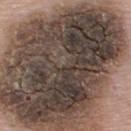Impression:
The lesion was tiled from a total-body skin photograph and was not biopsied.
Background:
A close-up tile cropped from a whole-body skin photograph, about 15 mm across. On the upper back. A male subject aged 53–57. The lesion's longest dimension is about 14.5 mm. Automated tile analysis of the lesion measured an average lesion color of about L≈38 a*≈11 b*≈18 (CIELAB), a lesion–skin lightness drop of about 18, and a normalized lesion–skin contrast near 14.5. The analysis additionally found a classifier nevus-likeness of about 0/100 and a detector confidence of about 50 out of 100 that the crop contains a lesion. This is a white-light tile.